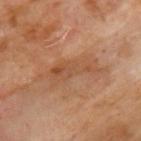Clinical impression: Part of a total-body skin-imaging series; this lesion was reviewed on a skin check and was not flagged for biopsy.